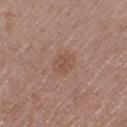Case summary:
• biopsy status: no biopsy performed (imaged during a skin exam)
• subject: male, in their mid-70s
• image-analysis metrics: a lesion area of about 4.5 mm², a shape eccentricity near 0.75, and two-axis asymmetry of about 0.25; a border-irregularity index near 3/10 and a within-lesion color-variation index near 2/10
• location: the leg
• size: ~3 mm (longest diameter)
• image source: ~15 mm tile from a whole-body skin photo
• tile lighting: white-light